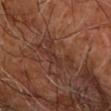follow-up = no biopsy performed (imaged during a skin exam); anatomic site = the right upper arm; subject = about 65 years old; lesion size = about 5.5 mm; imaging modality = 15 mm crop, total-body photography.